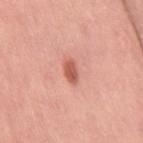The lesion was tiled from a total-body skin photograph and was not biopsied.
The lesion's longest dimension is about 3 mm.
Captured under white-light illumination.
The lesion is on the right thigh.
A region of skin cropped from a whole-body photographic capture, roughly 15 mm wide.
A female patient about 50 years old.